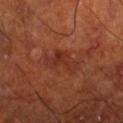Impression: The lesion was tiled from a total-body skin photograph and was not biopsied. Image and clinical context: A male patient, about 70 years old. A roughly 15 mm field-of-view crop from a total-body skin photograph. Longest diameter approximately 4.5 mm. Located on the right lower leg. The tile uses cross-polarized illumination.